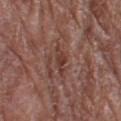Assessment:
Captured during whole-body skin photography for melanoma surveillance; the lesion was not biopsied.
Context:
A female patient, roughly 80 years of age. Cropped from a total-body skin-imaging series; the visible field is about 15 mm. The lesion is located on the right thigh. The recorded lesion diameter is about 3.5 mm.